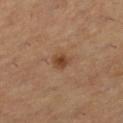This is a cross-polarized tile. Cropped from a whole-body photographic skin survey; the tile spans about 15 mm. The patient is a female roughly 55 years of age. Measured at roughly 2.5 mm in maximum diameter. The lesion is located on the left lower leg.